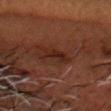Imaged during a routine full-body skin examination; the lesion was not biopsied and no histopathology is available. Located on the head or neck. A male patient, roughly 50 years of age. About 3 mm across. The tile uses cross-polarized illumination. A 15 mm close-up tile from a total-body photography series done for melanoma screening. The total-body-photography lesion software estimated an area of roughly 5.5 mm². And it measured a border-irregularity index near 2.5/10 and radial color variation of about 1.5. The analysis additionally found a nevus-likeness score of about 30/100 and a detector confidence of about 95 out of 100 that the crop contains a lesion.